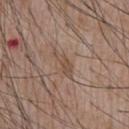Captured during whole-body skin photography for melanoma surveillance; the lesion was not biopsied.
A male patient, in their mid- to late 50s.
The lesion is located on the chest.
A close-up tile cropped from a whole-body skin photograph, about 15 mm across.